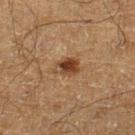The lesion was tiled from a total-body skin photograph and was not biopsied. On the left lower leg. The subject is a male in their mid- to late 60s. A 15 mm close-up tile from a total-body photography series done for melanoma screening.